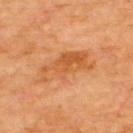- workup: total-body-photography surveillance lesion; no biopsy
- patient: male, aged around 60
- image: ~15 mm crop, total-body skin-cancer survey
- site: the upper back